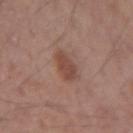Q: Was this lesion biopsied?
A: catalogued during a skin exam; not biopsied
Q: Who is the patient?
A: female, roughly 55 years of age
Q: Lesion location?
A: the left forearm
Q: What is the imaging modality?
A: total-body-photography crop, ~15 mm field of view